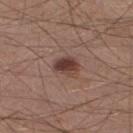Q: Was this lesion biopsied?
A: no biopsy performed (imaged during a skin exam)